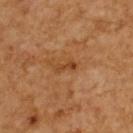{"biopsy_status": "not biopsied; imaged during a skin examination", "lesion_size": {"long_diameter_mm_approx": 3.0}, "image": {"source": "total-body photography crop", "field_of_view_mm": 15}, "site": "upper back", "lighting": "cross-polarized", "patient": {"sex": "male", "age_approx": 60}}